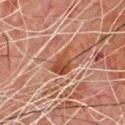Q: Was this lesion biopsied?
A: total-body-photography surveillance lesion; no biopsy
Q: Lesion location?
A: the chest
Q: What lighting was used for the tile?
A: cross-polarized
Q: Patient demographics?
A: male, roughly 80 years of age
Q: What kind of image is this?
A: total-body-photography crop, ~15 mm field of view
Q: What is the lesion's diameter?
A: ≈4 mm